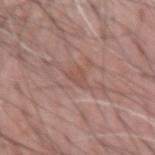Clinical impression: Captured during whole-body skin photography for melanoma surveillance; the lesion was not biopsied. Background: A male patient, in their 60s. This is a white-light tile. A roughly 15 mm field-of-view crop from a total-body skin photograph. The lesion's longest dimension is about 3 mm. From the right forearm.